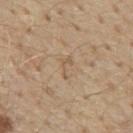notes: total-body-photography surveillance lesion; no biopsy
site: the front of the torso
automated lesion analysis: an eccentricity of roughly 0.85 and a symmetry-axis asymmetry near 0.65; an automated nevus-likeness rating near 0 out of 100
image: 15 mm crop, total-body photography
tile lighting: white-light illumination
patient: male, aged 68–72
diameter: about 2.5 mm